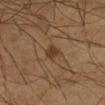Case summary:
• biopsy status: total-body-photography surveillance lesion; no biopsy
• image source: total-body-photography crop, ~15 mm field of view
• automated metrics: a lesion area of about 3.5 mm², an outline eccentricity of about 0.75 (0 = round, 1 = elongated), and a symmetry-axis asymmetry near 0.3; a lesion color around L≈37 a*≈18 b*≈31 in CIELAB and about 8 CIELAB-L* units darker than the surrounding skin; a detector confidence of about 100 out of 100 that the crop contains a lesion
• lighting: cross-polarized illumination
• anatomic site: the left forearm
• patient: male, approximately 60 years of age
• size: ~2.5 mm (longest diameter)